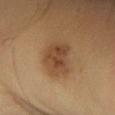Clinical impression:
Imaged during a routine full-body skin examination; the lesion was not biopsied and no histopathology is available.
Context:
A male subject, approximately 40 years of age. A region of skin cropped from a whole-body photographic capture, roughly 15 mm wide. Captured under cross-polarized illumination. The total-body-photography lesion software estimated an area of roughly 12 mm², an outline eccentricity of about 0.55 (0 = round, 1 = elongated), and a shape-asymmetry score of about 0.25 (0 = symmetric). And it measured a lesion color around L≈45 a*≈19 b*≈32 in CIELAB. Measured at roughly 4 mm in maximum diameter. From the left lower leg.